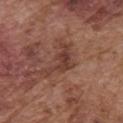biopsy status: catalogued during a skin exam; not biopsied | acquisition: ~15 mm crop, total-body skin-cancer survey | patient: male, aged 73 to 77 | automated metrics: a lesion–skin lightness drop of about 8 | diameter: about 5.5 mm | site: the front of the torso | tile lighting: white-light.